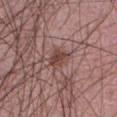Recorded during total-body skin imaging; not selected for excision or biopsy.
A male subject aged around 60.
Cropped from a whole-body photographic skin survey; the tile spans about 15 mm.
On the abdomen.
Imaged with white-light lighting.